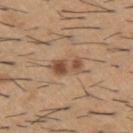{
  "biopsy_status": "not biopsied; imaged during a skin examination",
  "patient": {
    "sex": "male",
    "age_approx": 60
  },
  "image": {
    "source": "total-body photography crop",
    "field_of_view_mm": 15
  },
  "site": "upper back",
  "lighting": "white-light"
}